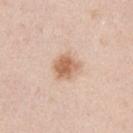Part of a total-body skin-imaging series; this lesion was reviewed on a skin check and was not flagged for biopsy. This image is a 15 mm lesion crop taken from a total-body photograph. A male subject, aged 38 to 42. From the arm. Automated image analysis of the tile measured an eccentricity of roughly 0.45 and two-axis asymmetry of about 0.25. The software also gave a border-irregularity index near 2.5/10, a within-lesion color-variation index near 3.5/10, and a peripheral color-asymmetry measure near 1. Measured at roughly 3.5 mm in maximum diameter.